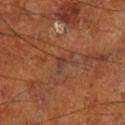Part of a total-body skin-imaging series; this lesion was reviewed on a skin check and was not flagged for biopsy. Located on the leg. A 15 mm crop from a total-body photograph taken for skin-cancer surveillance. A male patient roughly 65 years of age.